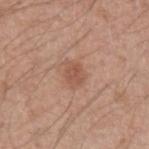{
  "automated_metrics": {
    "eccentricity": 0.7,
    "shape_asymmetry": 0.2,
    "nevus_likeness_0_100": 50
  },
  "patient": {
    "sex": "male",
    "age_approx": 55
  },
  "lesion_size": {
    "long_diameter_mm_approx": 3.0
  },
  "lighting": "white-light",
  "image": {
    "source": "total-body photography crop",
    "field_of_view_mm": 15
  }
}